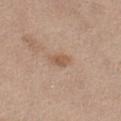biopsy status: no biopsy performed (imaged during a skin exam) | acquisition: 15 mm crop, total-body photography | body site: the abdomen | automated metrics: a footprint of about 3.5 mm²; a normalized lesion–skin contrast near 6.5; a nevus-likeness score of about 5/100 and a lesion-detection confidence of about 100/100 | subject: female, in their mid- to late 60s | lesion diameter: ≈2.5 mm.